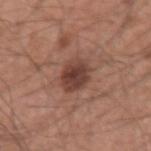Impression: The lesion was photographed on a routine skin check and not biopsied; there is no pathology result. Clinical summary: A 15 mm crop from a total-body photograph taken for skin-cancer surveillance. Captured under white-light illumination. Located on the left upper arm. The subject is a male aged around 60.